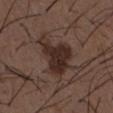The lesion was photographed on a routine skin check and not biopsied; there is no pathology result. Approximately 6 mm at its widest. This is a white-light tile. The lesion is on the chest. Cropped from a whole-body photographic skin survey; the tile spans about 15 mm. A male subject, in their 50s. Automated image analysis of the tile measured an outline eccentricity of about 0.7 (0 = round, 1 = elongated) and a shape-asymmetry score of about 0.5 (0 = symmetric). And it measured a lesion color around L≈28 a*≈16 b*≈20 in CIELAB and a lesion–skin lightness drop of about 10.